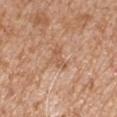Assessment: The lesion was tiled from a total-body skin photograph and was not biopsied. Image and clinical context: Located on the right upper arm. This is a white-light tile. A male patient aged 63 to 67. Cropped from a whole-body photographic skin survey; the tile spans about 15 mm.